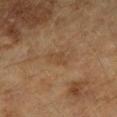Assessment: This lesion was catalogued during total-body skin photography and was not selected for biopsy. Background: The lesion-visualizer software estimated a lesion color around L≈41 a*≈16 b*≈30 in CIELAB and a normalized border contrast of about 5. The analysis additionally found a lesion-detection confidence of about 100/100. A 15 mm close-up tile from a total-body photography series done for melanoma screening. Imaged with cross-polarized lighting. A female subject roughly 60 years of age. From the left forearm.